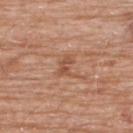  biopsy_status: not biopsied; imaged during a skin examination
  lighting: white-light
  patient:
    sex: male
    age_approx: 80
  automated_metrics:
    area_mm2_approx: 4.5
    shape_asymmetry: 0.4
    nevus_likeness_0_100: 0
    lesion_detection_confidence_0_100: 100
  lesion_size:
    long_diameter_mm_approx: 3.0
  image:
    source: total-body photography crop
    field_of_view_mm: 15
  site: upper back